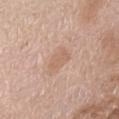Case summary:
• notes: catalogued during a skin exam; not biopsied
• patient: female, in their mid-70s
• anatomic site: the front of the torso
• illumination: white-light
• image: ~15 mm crop, total-body skin-cancer survey